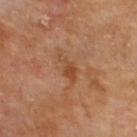biopsy status=imaged on a skin check; not biopsied
location=the upper back
image source=~15 mm crop, total-body skin-cancer survey
patient=male, aged around 70